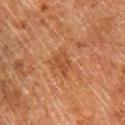  biopsy_status: not biopsied; imaged during a skin examination
  patient:
    sex: male
    age_approx: 80
  automated_metrics:
    eccentricity: 0.6
    shape_asymmetry: 0.25
    cielab_L: 38
    cielab_a: 21
    cielab_b: 31
    vs_skin_darker_L: 6.0
    vs_skin_contrast_norm: 5.5
    color_variation_0_10: 2.0
    peripheral_color_asymmetry: 1.0
  site: leg
  lighting: cross-polarized
  lesion_size:
    long_diameter_mm_approx: 2.5
  image:
    source: total-body photography crop
    field_of_view_mm: 15